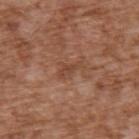The lesion was tiled from a total-body skin photograph and was not biopsied. The lesion is located on the back. A region of skin cropped from a whole-body photographic capture, roughly 15 mm wide. The patient is a male in their mid- to late 70s.